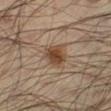No biopsy was performed on this lesion — it was imaged during a full skin examination and was not determined to be concerning.
Measured at roughly 3.5 mm in maximum diameter.
From the left lower leg.
The patient is a male aged approximately 35.
A 15 mm crop from a total-body photograph taken for skin-cancer surveillance.